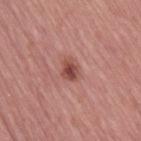Context: A female subject, aged 63–67. The lesion is on the right thigh. A close-up tile cropped from a whole-body skin photograph, about 15 mm across.